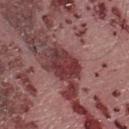Impression:
The lesion was tiled from a total-body skin photograph and was not biopsied.
Context:
On the right lower leg. A female subject about 20 years old. Measured at roughly 4.5 mm in maximum diameter. A close-up tile cropped from a whole-body skin photograph, about 15 mm across.